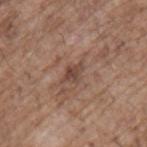Captured under white-light illumination.
Automated tile analysis of the lesion measured a nevus-likeness score of about 0/100 and a lesion-detection confidence of about 100/100.
A close-up tile cropped from a whole-body skin photograph, about 15 mm across.
From the left upper arm.
The subject is a male in their 70s.